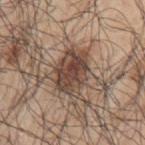biopsy status: total-body-photography surveillance lesion; no biopsy | subject: male, aged approximately 70 | site: the left upper arm | acquisition: 15 mm crop, total-body photography.